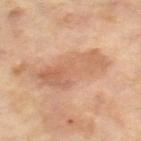Notes:
- notes — catalogued during a skin exam; not biopsied
- body site — the right thigh
- patient — female, aged 58–62
- image — ~15 mm crop, total-body skin-cancer survey